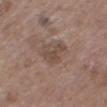Assessment:
The lesion was tiled from a total-body skin photograph and was not biopsied.
Background:
A male subject, approximately 75 years of age. A 15 mm close-up tile from a total-body photography series done for melanoma screening. From the right thigh.